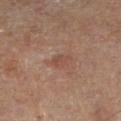Captured during whole-body skin photography for melanoma surveillance; the lesion was not biopsied.
A region of skin cropped from a whole-body photographic capture, roughly 15 mm wide.
The lesion is on the leg.
A male subject, aged approximately 65.